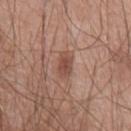Clinical impression: No biopsy was performed on this lesion — it was imaged during a full skin examination and was not determined to be concerning. Acquisition and patient details: An algorithmic analysis of the crop reported an area of roughly 4.5 mm², an eccentricity of roughly 0.8, and a shape-asymmetry score of about 0.3 (0 = symmetric). Cropped from a whole-body photographic skin survey; the tile spans about 15 mm. The recorded lesion diameter is about 3 mm. The subject is a male in their mid- to late 60s. Captured under white-light illumination. From the chest.